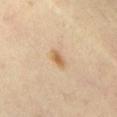workup — imaged on a skin check; not biopsied | lighting — cross-polarized | anatomic site — the abdomen | diameter — about 2.5 mm | patient — male, roughly 45 years of age | imaging modality — total-body-photography crop, ~15 mm field of view.